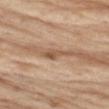This lesion was catalogued during total-body skin photography and was not selected for biopsy.
This image is a 15 mm lesion crop taken from a total-body photograph.
From the left thigh.
A male patient roughly 70 years of age.
The lesion's longest dimension is about 3.5 mm.
Captured under white-light illumination.